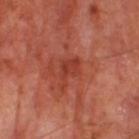Clinical impression: Imaged during a routine full-body skin examination; the lesion was not biopsied and no histopathology is available. Background: Approximately 3.5 mm at its widest. From the right thigh. A male subject, in their 70s. Imaged with cross-polarized lighting. The lesion-visualizer software estimated a footprint of about 5.5 mm² and an eccentricity of roughly 0.75. It also reported a lesion-to-skin contrast of about 6 (normalized; higher = more distinct). A 15 mm close-up tile from a total-body photography series done for melanoma screening.